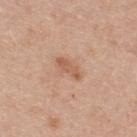  patient:
    sex: female
    age_approx: 40
  lesion_size:
    long_diameter_mm_approx: 3.5
  lighting: white-light
  site: upper back
  automated_metrics:
    cielab_L: 58
    cielab_a: 21
    cielab_b: 33
    border_irregularity_0_10: 3.5
  image:
    source: total-body photography crop
    field_of_view_mm: 15